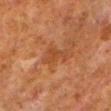The lesion was photographed on a routine skin check and not biopsied; there is no pathology result.
This image is a 15 mm lesion crop taken from a total-body photograph.
This is a cross-polarized tile.
The lesion is on the left lower leg.
The patient is a male aged 78 to 82.
Automated image analysis of the tile measured an area of roughly 4.5 mm², an eccentricity of roughly 0.8, and a symmetry-axis asymmetry near 0.55. The software also gave a lesion color around L≈36 a*≈22 b*≈32 in CIELAB, roughly 5 lightness units darker than nearby skin, and a lesion-to-skin contrast of about 5.5 (normalized; higher = more distinct).